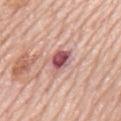Q: Is there a histopathology result?
A: imaged on a skin check; not biopsied
Q: Illumination type?
A: white-light
Q: What did automated image analysis measure?
A: an area of roughly 5.5 mm², an eccentricity of roughly 0.75, and a symmetry-axis asymmetry near 0.25; a mean CIELAB color near L≈54 a*≈29 b*≈20 and roughly 16 lightness units darker than nearby skin; a classifier nevus-likeness of about 5/100 and a lesion-detection confidence of about 100/100
Q: Lesion size?
A: ≈3.5 mm
Q: What are the patient's age and sex?
A: male, in their 80s
Q: What is the imaging modality?
A: ~15 mm tile from a whole-body skin photo
Q: Where on the body is the lesion?
A: the mid back